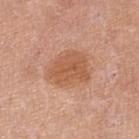The lesion was tiled from a total-body skin photograph and was not biopsied. A female subject roughly 65 years of age. About 4.5 mm across. Imaged with white-light lighting. The lesion-visualizer software estimated a shape-asymmetry score of about 0.2 (0 = symmetric). And it measured a mean CIELAB color near L≈56 a*≈24 b*≈35, a lesion–skin lightness drop of about 9, and a lesion-to-skin contrast of about 7 (normalized; higher = more distinct). And it measured a border-irregularity rating of about 2.5/10, a within-lesion color-variation index near 2.5/10, and peripheral color asymmetry of about 1. The software also gave a classifier nevus-likeness of about 30/100. Cropped from a total-body skin-imaging series; the visible field is about 15 mm. From the left lower leg.